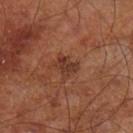No biopsy was performed on this lesion — it was imaged during a full skin examination and was not determined to be concerning. Cropped from a whole-body photographic skin survey; the tile spans about 15 mm. The lesion is on the leg. The subject is a male aged around 70. The recorded lesion diameter is about 2.5 mm. The tile uses cross-polarized illumination.